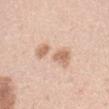Imaged during a routine full-body skin examination; the lesion was not biopsied and no histopathology is available. Automated tile analysis of the lesion measured an average lesion color of about L≈69 a*≈18 b*≈31 (CIELAB). The analysis additionally found a border-irregularity rating of about 4.5/10, a within-lesion color-variation index near 3.5/10, and peripheral color asymmetry of about 1.5. Measured at roughly 6.5 mm in maximum diameter. The subject is a male aged around 45. The lesion is on the right upper arm. Imaged with white-light lighting. This image is a 15 mm lesion crop taken from a total-body photograph.